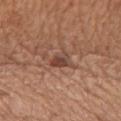site: mid back
lesion_size:
  long_diameter_mm_approx: 2.5
lighting: white-light
patient:
  sex: male
  age_approx: 65
image:
  source: total-body photography crop
  field_of_view_mm: 15